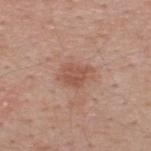Part of a total-body skin-imaging series; this lesion was reviewed on a skin check and was not flagged for biopsy. A male subject, in their 40s. A 15 mm close-up extracted from a 3D total-body photography capture. Measured at roughly 3.5 mm in maximum diameter. The lesion is located on the upper back.